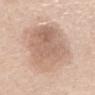| feature | finding |
|---|---|
| patient | male, in their 60s |
| image | ~15 mm crop, total-body skin-cancer survey |
| anatomic site | the mid back |